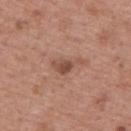Assessment: No biopsy was performed on this lesion — it was imaged during a full skin examination and was not determined to be concerning. Context: The lesion is located on the upper back. A male subject in their mid- to late 60s. A 15 mm crop from a total-body photograph taken for skin-cancer surveillance. Measured at roughly 4 mm in maximum diameter. Captured under white-light illumination.